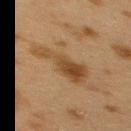<lesion>
<biopsy_status>not biopsied; imaged during a skin examination</biopsy_status>
<image>
  <source>total-body photography crop</source>
  <field_of_view_mm>15</field_of_view_mm>
</image>
<lighting>cross-polarized</lighting>
<lesion_size>
  <long_diameter_mm_approx>6.0</long_diameter_mm_approx>
</lesion_size>
<site>upper back</site>
<patient>
  <sex>female</sex>
  <age_approx>40</age_approx>
</patient>
</lesion>